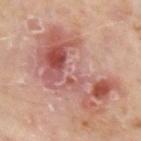notes = total-body-photography surveillance lesion; no biopsy
subject = male, about 75 years old
lesion size = about 10.5 mm
site = the arm
image source = 15 mm crop, total-body photography